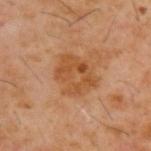Findings:
• notes · imaged on a skin check; not biopsied
• patient · male, aged 58 to 62
• lighting · cross-polarized illumination
• body site · the upper back
• size · ≈4.5 mm
• TBP lesion metrics · border irregularity of about 2.5 on a 0–10 scale, internal color variation of about 4.5 on a 0–10 scale, and peripheral color asymmetry of about 1.5
• acquisition · ~15 mm crop, total-body skin-cancer survey